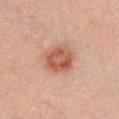notes: no biopsy performed (imaged during a skin exam); image source: total-body-photography crop, ~15 mm field of view; diameter: about 3.5 mm; tile lighting: white-light illumination; body site: the chest; patient: female, approximately 25 years of age.